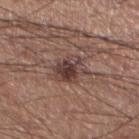Recorded during total-body skin imaging; not selected for excision or biopsy. A roughly 15 mm field-of-view crop from a total-body skin photograph. A male subject, roughly 35 years of age. An algorithmic analysis of the crop reported a lesion color around L≈40 a*≈18 b*≈20 in CIELAB and a normalized lesion–skin contrast near 9. Located on the left lower leg. Imaged with white-light lighting. Longest diameter approximately 4 mm.